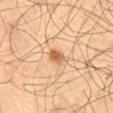Findings:
• biopsy status: catalogued during a skin exam; not biopsied
• size: ~2.5 mm (longest diameter)
• image-analysis metrics: a footprint of about 3.5 mm², a shape eccentricity near 0.8, and two-axis asymmetry of about 0.25; a lesion–skin lightness drop of about 11 and a normalized border contrast of about 8; border irregularity of about 2.5 on a 0–10 scale and a within-lesion color-variation index near 4.5/10
• patient: male, in their 70s
• tile lighting: cross-polarized illumination
• imaging modality: ~15 mm crop, total-body skin-cancer survey
• anatomic site: the right thigh